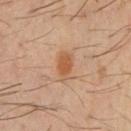Recorded during total-body skin imaging; not selected for excision or biopsy. A close-up tile cropped from a whole-body skin photograph, about 15 mm across. The lesion is located on the front of the torso. A male subject aged 58–62.